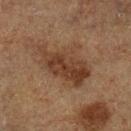biopsy_status: not biopsied; imaged during a skin examination
patient:
  sex: male
  age_approx: 75
lighting: cross-polarized
image:
  source: total-body photography crop
  field_of_view_mm: 15
site: left lower leg
lesion_size:
  long_diameter_mm_approx: 7.5
automated_metrics:
  area_mm2_approx: 20.0
  eccentricity: 0.8
  shape_asymmetry: 0.3
  vs_skin_darker_L: 8.0
  vs_skin_contrast_norm: 8.0
  border_irregularity_0_10: 4.5
  color_variation_0_10: 5.0
  peripheral_color_asymmetry: 2.0